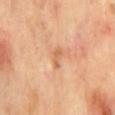Q: Was a biopsy performed?
A: no biopsy performed (imaged during a skin exam)
Q: What kind of image is this?
A: ~15 mm crop, total-body skin-cancer survey
Q: What is the anatomic site?
A: the mid back